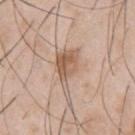Assessment: The lesion was photographed on a routine skin check and not biopsied; there is no pathology result. Clinical summary: The recorded lesion diameter is about 6 mm. Automated image analysis of the tile measured a border-irregularity index near 6.5/10, internal color variation of about 5 on a 0–10 scale, and peripheral color asymmetry of about 2. From the left upper arm. A lesion tile, about 15 mm wide, cut from a 3D total-body photograph. Imaged with white-light lighting. The subject is a male in their mid-50s.